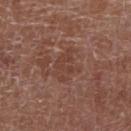Imaged during a routine full-body skin examination; the lesion was not biopsied and no histopathology is available.
The lesion is located on the arm.
About 4 mm across.
Cropped from a whole-body photographic skin survey; the tile spans about 15 mm.
This is a white-light tile.
A female subject aged approximately 80.
Automated image analysis of the tile measured a footprint of about 7 mm², an outline eccentricity of about 0.75 (0 = round, 1 = elongated), and a shape-asymmetry score of about 0.35 (0 = symmetric). The software also gave a border-irregularity rating of about 7.5/10, a color-variation rating of about 1/10, and a peripheral color-asymmetry measure near 0.5.